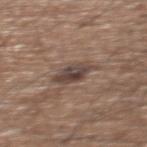* notes: no biopsy performed (imaged during a skin exam)
* patient: male, in their mid-70s
* imaging modality: ~15 mm crop, total-body skin-cancer survey
* diameter: about 4 mm
* anatomic site: the upper back